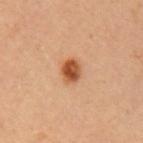| key | value |
|---|---|
| workup | catalogued during a skin exam; not biopsied |
| acquisition | 15 mm crop, total-body photography |
| anatomic site | the left upper arm |
| patient | female, in their mid-30s |